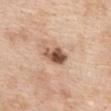<tbp_lesion>
  <biopsy_status>not biopsied; imaged during a skin examination</biopsy_status>
  <automated_metrics>
    <eccentricity>0.85</eccentricity>
    <shape_asymmetry>0.2</shape_asymmetry>
    <border_irregularity_0_10>2.0</border_irregularity_0_10>
    <color_variation_0_10>8.5</color_variation_0_10>
    <peripheral_color_asymmetry>4.0</peripheral_color_asymmetry>
    <nevus_likeness_0_100>75</nevus_likeness_0_100>
    <lesion_detection_confidence_0_100>100</lesion_detection_confidence_0_100>
  </automated_metrics>
  <lighting>white-light</lighting>
  <image>
    <source>total-body photography crop</source>
    <field_of_view_mm>15</field_of_view_mm>
  </image>
  <lesion_size>
    <long_diameter_mm_approx>3.5</long_diameter_mm_approx>
  </lesion_size>
  <site>chest</site>
  <patient>
    <sex>female</sex>
    <age_approx>40</age_approx>
  </patient>
</tbp_lesion>